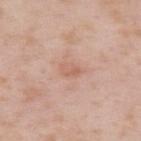anatomic site=the upper back | image source=~15 mm tile from a whole-body skin photo | patient=male, approximately 55 years of age.